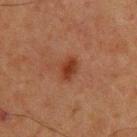Clinical impression:
Part of a total-body skin-imaging series; this lesion was reviewed on a skin check and was not flagged for biopsy.
Image and clinical context:
Captured under cross-polarized illumination. The patient is a male aged around 60. A close-up tile cropped from a whole-body skin photograph, about 15 mm across. About 2.5 mm across. Automated image analysis of the tile measured a footprint of about 4 mm² and a shape eccentricity near 0.7. It also reported a lesion color around L≈29 a*≈22 b*≈27 in CIELAB, about 8 CIELAB-L* units darker than the surrounding skin, and a lesion-to-skin contrast of about 8.5 (normalized; higher = more distinct). And it measured a nevus-likeness score of about 95/100 and a detector confidence of about 100 out of 100 that the crop contains a lesion. From the upper back.